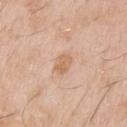– notes · imaged on a skin check; not biopsied
– imaging modality · ~15 mm crop, total-body skin-cancer survey
– patient · male, in their 50s
– diameter · ≈2.5 mm
– illumination · white-light
– anatomic site · the left upper arm